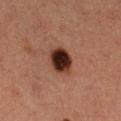Clinical impression: Recorded during total-body skin imaging; not selected for excision or biopsy. Clinical summary: On the left thigh. Imaged with cross-polarized lighting. The lesion's longest dimension is about 3.5 mm. A lesion tile, about 15 mm wide, cut from a 3D total-body photograph. A female patient, aged around 40.